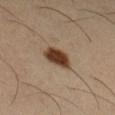No biopsy was performed on this lesion — it was imaged during a full skin examination and was not determined to be concerning.
A male subject, aged approximately 40.
The lesion is located on the left upper arm.
Cropped from a total-body skin-imaging series; the visible field is about 15 mm.